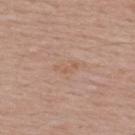The lesion was tiled from a total-body skin photograph and was not biopsied.
Automated tile analysis of the lesion measured a border-irregularity index near 6/10 and radial color variation of about 0.
A lesion tile, about 15 mm wide, cut from a 3D total-body photograph.
From the upper back.
About 2.5 mm across.
Captured under white-light illumination.
The subject is a male aged 38–42.